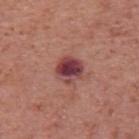The lesion was tiled from a total-body skin photograph and was not biopsied. About 3 mm across. Located on the upper back. Captured under white-light illumination. A roughly 15 mm field-of-view crop from a total-body skin photograph. A male subject aged around 70.